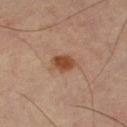The lesion was tiled from a total-body skin photograph and was not biopsied. The lesion's longest dimension is about 3 mm. A 15 mm close-up extracted from a 3D total-body photography capture. On the right thigh. An algorithmic analysis of the crop reported a lesion area of about 5.5 mm², an eccentricity of roughly 0.65, and a symmetry-axis asymmetry near 0.25. The software also gave a normalized border contrast of about 9.5. It also reported border irregularity of about 2 on a 0–10 scale, a color-variation rating of about 2.5/10, and radial color variation of about 1. It also reported an automated nevus-likeness rating near 95 out of 100 and lesion-presence confidence of about 100/100. Imaged with cross-polarized lighting.